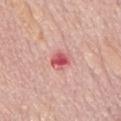biopsy_status: not biopsied; imaged during a skin examination
lighting: white-light
site: mid back
lesion_size:
  long_diameter_mm_approx: 2.5
image:
  source: total-body photography crop
  field_of_view_mm: 15
automated_metrics:
  cielab_L: 57
  cielab_a: 36
  cielab_b: 24
  vs_skin_darker_L: 14.0
  border_irregularity_0_10: 2.5
  color_variation_0_10: 4.5
  peripheral_color_asymmetry: 1.5
  nevus_likeness_0_100: 0
  lesion_detection_confidence_0_100: 100
patient:
  sex: male
  age_approx: 75